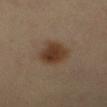notes: no biopsy performed (imaged during a skin exam) | patient: female, in their 40s | imaging modality: ~15 mm tile from a whole-body skin photo | anatomic site: the left lower leg.